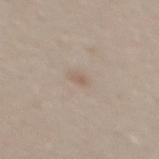The lesion was tiled from a total-body skin photograph and was not biopsied.
A 15 mm crop from a total-body photograph taken for skin-cancer surveillance.
Located on the mid back.
The lesion's longest dimension is about 1.5 mm.
Automated tile analysis of the lesion measured a lesion area of about 1.5 mm² and a shape eccentricity near 0.75. It also reported a border-irregularity rating of about 2/10 and a within-lesion color-variation index near 0/10. The software also gave an automated nevus-likeness rating near 0 out of 100 and a lesion-detection confidence of about 100/100.
The subject is a male roughly 30 years of age.